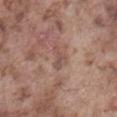The lesion was photographed on a routine skin check and not biopsied; there is no pathology result.
A male patient, aged around 75.
The lesion is on the abdomen.
Captured under white-light illumination.
An algorithmic analysis of the crop reported an area of roughly 3 mm², a shape eccentricity near 0.9, and a shape-asymmetry score of about 0.65 (0 = symmetric). It also reported border irregularity of about 7.5 on a 0–10 scale, internal color variation of about 0 on a 0–10 scale, and a peripheral color-asymmetry measure near 0.
A close-up tile cropped from a whole-body skin photograph, about 15 mm across.
About 3 mm across.